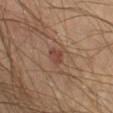Impression:
The lesion was tiled from a total-body skin photograph and was not biopsied.
Clinical summary:
The lesion-visualizer software estimated an eccentricity of roughly 0.7. The software also gave a lesion color around L≈42 a*≈22 b*≈25 in CIELAB, a lesion–skin lightness drop of about 8, and a lesion-to-skin contrast of about 6.5 (normalized; higher = more distinct). The analysis additionally found a border-irregularity index near 3/10 and internal color variation of about 2 on a 0–10 scale. And it measured an automated nevus-likeness rating near 5 out of 100 and a detector confidence of about 100 out of 100 that the crop contains a lesion. From the left lower leg. Approximately 2.5 mm at its widest. The tile uses cross-polarized illumination. A region of skin cropped from a whole-body photographic capture, roughly 15 mm wide. A male patient roughly 45 years of age.